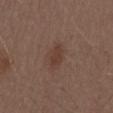Findings:
* follow-up — total-body-photography surveillance lesion; no biopsy
* lighting — white-light
* automated metrics — a footprint of about 5.5 mm², a shape eccentricity near 0.8, and a symmetry-axis asymmetry near 0.25
* patient — male, aged 68–72
* acquisition — ~15 mm tile from a whole-body skin photo
* body site — the mid back